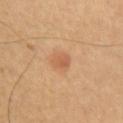Captured during whole-body skin photography for melanoma surveillance; the lesion was not biopsied.
The subject is a male aged approximately 60.
The lesion is located on the right upper arm.
A roughly 15 mm field-of-view crop from a total-body skin photograph.
Imaged with cross-polarized lighting.